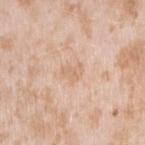Assessment: Part of a total-body skin-imaging series; this lesion was reviewed on a skin check and was not flagged for biopsy. Acquisition and patient details: The tile uses white-light illumination. About 2.5 mm across. Cropped from a total-body skin-imaging series; the visible field is about 15 mm. Located on the right upper arm. A female patient aged approximately 25.